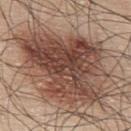The lesion was photographed on a routine skin check and not biopsied; there is no pathology result. A male patient, approximately 45 years of age. Longest diameter approximately 9.5 mm. A 15 mm close-up tile from a total-body photography series done for melanoma screening. On the upper back. Automated image analysis of the tile measured a footprint of about 45 mm², an eccentricity of roughly 0.6, and a symmetry-axis asymmetry near 0.5. Captured under white-light illumination.